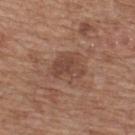<case>
  <biopsy_status>not biopsied; imaged during a skin examination</biopsy_status>
  <lighting>white-light</lighting>
  <image>
    <source>total-body photography crop</source>
    <field_of_view_mm>15</field_of_view_mm>
  </image>
  <patient>
    <sex>male</sex>
    <age_approx>65</age_approx>
  </patient>
  <site>upper back</site>
</case>